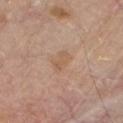The lesion was tiled from a total-body skin photograph and was not biopsied. Cropped from a total-body skin-imaging series; the visible field is about 15 mm. A male subject aged around 80. From the chest.